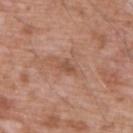workup: imaged on a skin check; not biopsied
site: the chest
lighting: white-light
lesion diameter: about 2.5 mm
subject: male, aged 58 to 62
automated metrics: a symmetry-axis asymmetry near 0.25; about 8 CIELAB-L* units darker than the surrounding skin and a normalized lesion–skin contrast near 6; a nevus-likeness score of about 0/100
imaging modality: ~15 mm tile from a whole-body skin photo